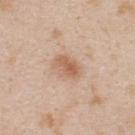The lesion was photographed on a routine skin check and not biopsied; there is no pathology result.
The tile uses white-light illumination.
A male patient, about 25 years old.
About 3.5 mm across.
A region of skin cropped from a whole-body photographic capture, roughly 15 mm wide.
From the back.